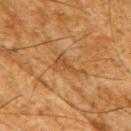notes: imaged on a skin check; not biopsied
patient: male, aged approximately 65
lesion diameter: ~4 mm (longest diameter)
lighting: cross-polarized
location: the right upper arm
automated metrics: a lesion area of about 5.5 mm², an eccentricity of roughly 0.85, and a shape-asymmetry score of about 0.6 (0 = symmetric); an average lesion color of about L≈40 a*≈18 b*≈34 (CIELAB), a lesion–skin lightness drop of about 6, and a lesion-to-skin contrast of about 5 (normalized; higher = more distinct); an automated nevus-likeness rating near 0 out of 100 and lesion-presence confidence of about 60/100
image: ~15 mm tile from a whole-body skin photo